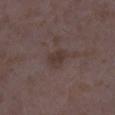workup: no biopsy performed (imaged during a skin exam)
subject: female, about 35 years old
image-analysis metrics: a footprint of about 4.5 mm², an eccentricity of roughly 0.7, and two-axis asymmetry of about 0.25
size: ≈3 mm
acquisition: ~15 mm crop, total-body skin-cancer survey
location: the right lower leg